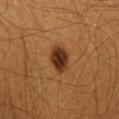Part of a total-body skin-imaging series; this lesion was reviewed on a skin check and was not flagged for biopsy. Automated tile analysis of the lesion measured a lesion area of about 7 mm², a shape eccentricity near 0.75, and a shape-asymmetry score of about 0.25 (0 = symmetric). A male subject aged around 60. This image is a 15 mm lesion crop taken from a total-body photograph. Located on the chest.